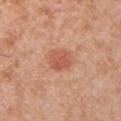notes: no biopsy performed (imaged during a skin exam)
tile lighting: white-light
image source: ~15 mm crop, total-body skin-cancer survey
subject: male, in their 30s
body site: the chest
automated lesion analysis: a footprint of about 6.5 mm², an outline eccentricity of about 0.55 (0 = round, 1 = elongated), and a symmetry-axis asymmetry near 0.25; lesion-presence confidence of about 100/100